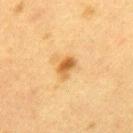Imaged during a routine full-body skin examination; the lesion was not biopsied and no histopathology is available. A female patient, aged 38 to 42. Captured under cross-polarized illumination. A region of skin cropped from a whole-body photographic capture, roughly 15 mm wide. The recorded lesion diameter is about 2.5 mm. The lesion is located on the mid back.